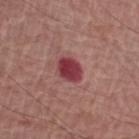Impression:
The lesion was photographed on a routine skin check and not biopsied; there is no pathology result.
Background:
A close-up tile cropped from a whole-body skin photograph, about 15 mm across. Approximately 3 mm at its widest. Automated image analysis of the tile measured an area of roughly 6 mm² and a shape eccentricity near 0.65. It also reported about 14 CIELAB-L* units darker than the surrounding skin and a lesion-to-skin contrast of about 11.5 (normalized; higher = more distinct). The software also gave border irregularity of about 1.5 on a 0–10 scale and a peripheral color-asymmetry measure near 1. The lesion is located on the left upper arm. A male subject aged 63–67. Captured under white-light illumination.